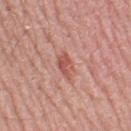notes=no biopsy performed (imaged during a skin exam); image=total-body-photography crop, ~15 mm field of view; patient=male, aged 68 to 72; body site=the left thigh.